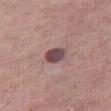The lesion was tiled from a total-body skin photograph and was not biopsied. A close-up tile cropped from a whole-body skin photograph, about 15 mm across. From the right thigh. About 3 mm across. A male subject, aged 73 to 77. The tile uses white-light illumination.